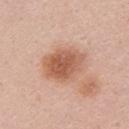biopsy_status: not biopsied; imaged during a skin examination
patient:
  sex: female
  age_approx: 20
lesion_size:
  long_diameter_mm_approx: 5.0
image:
  source: total-body photography crop
  field_of_view_mm: 15
automated_metrics:
  cielab_L: 58
  cielab_a: 23
  cielab_b: 32
  vs_skin_darker_L: 12.0
  vs_skin_contrast_norm: 8.5
site: back
lighting: white-light